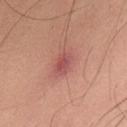The lesion was photographed on a routine skin check and not biopsied; there is no pathology result. A lesion tile, about 15 mm wide, cut from a 3D total-body photograph. Measured at roughly 3 mm in maximum diameter. This is a white-light tile. A male subject, aged 58–62. Automated tile analysis of the lesion measured a mean CIELAB color near L≈52 a*≈29 b*≈25, a lesion–skin lightness drop of about 9, and a normalized lesion–skin contrast near 7. On the upper back.